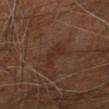{"biopsy_status": "not biopsied; imaged during a skin examination", "patient": {"sex": "male", "age_approx": 60}, "lesion_size": {"long_diameter_mm_approx": 3.0}, "lighting": "cross-polarized", "site": "leg", "image": {"source": "total-body photography crop", "field_of_view_mm": 15}}